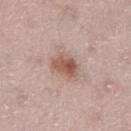– workup — no biopsy performed (imaged during a skin exam)
– patient — male, roughly 65 years of age
– acquisition — ~15 mm tile from a whole-body skin photo
– illumination — white-light
– size — about 3.5 mm
– location — the leg